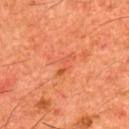  biopsy_status: not biopsied; imaged during a skin examination
  lesion_size:
    long_diameter_mm_approx: 3.0
  image:
    source: total-body photography crop
    field_of_view_mm: 15
  patient:
    sex: male
    age_approx: 60
  automated_metrics:
    area_mm2_approx: 3.0
    eccentricity: 0.9
    vs_skin_darker_L: 6.0
    vs_skin_contrast_norm: 4.5
    color_variation_0_10: 0.0
    peripheral_color_asymmetry: 0.0
  site: upper back
  lighting: cross-polarized